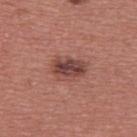| key | value |
|---|---|
| follow-up | no biopsy performed (imaged during a skin exam) |
| subject | male, in their 40s |
| size | ~4 mm (longest diameter) |
| body site | the upper back |
| imaging modality | 15 mm crop, total-body photography |
| illumination | white-light illumination |
| image-analysis metrics | an area of roughly 9 mm², an outline eccentricity of about 0.75 (0 = round, 1 = elongated), and a symmetry-axis asymmetry near 0.25; an average lesion color of about L≈44 a*≈24 b*≈23 (CIELAB), about 11 CIELAB-L* units darker than the surrounding skin, and a normalized lesion–skin contrast near 8.5; border irregularity of about 2.5 on a 0–10 scale and radial color variation of about 2 |